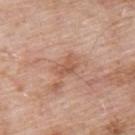Assessment: The lesion was tiled from a total-body skin photograph and was not biopsied. Clinical summary: This is a white-light tile. A close-up tile cropped from a whole-body skin photograph, about 15 mm across. Located on the upper back. A male subject, aged approximately 55. The total-body-photography lesion software estimated a nevus-likeness score of about 0/100 and lesion-presence confidence of about 100/100. About 3 mm across.